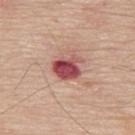biopsy status = no biopsy performed (imaged during a skin exam); lesion diameter = ≈3.5 mm; patient = male, in their 70s; location = the upper back; image source = total-body-photography crop, ~15 mm field of view; automated metrics = an automated nevus-likeness rating near 0 out of 100 and a detector confidence of about 100 out of 100 that the crop contains a lesion.